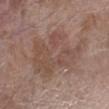Impression: Part of a total-body skin-imaging series; this lesion was reviewed on a skin check and was not flagged for biopsy. Clinical summary: The tile uses white-light illumination. The subject is a male about 60 years old. This image is a 15 mm lesion crop taken from a total-body photograph. From the left forearm. The total-body-photography lesion software estimated a footprint of about 32 mm², an outline eccentricity of about 0.85 (0 = round, 1 = elongated), and a symmetry-axis asymmetry near 0.35. And it measured a lesion color around L≈49 a*≈17 b*≈24 in CIELAB. The analysis additionally found a border-irregularity index near 5.5/10, internal color variation of about 4 on a 0–10 scale, and a peripheral color-asymmetry measure near 1. The software also gave an automated nevus-likeness rating near 0 out of 100 and a lesion-detection confidence of about 100/100. Approximately 10 mm at its widest.